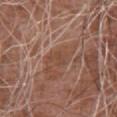{
  "biopsy_status": "not biopsied; imaged during a skin examination",
  "lighting": "white-light",
  "automated_metrics": {
    "area_mm2_approx": 4.0,
    "eccentricity": 0.65,
    "shape_asymmetry": 0.4,
    "nevus_likeness_0_100": 0
  },
  "patient": {
    "sex": "male",
    "age_approx": 75
  },
  "image": {
    "source": "total-body photography crop",
    "field_of_view_mm": 15
  },
  "site": "chest"
}